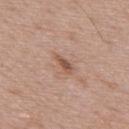automated_metrics:
  cielab_L: 53
  cielab_a: 20
  cielab_b: 29
  vs_skin_darker_L: 10.0
  vs_skin_contrast_norm: 7.5
lighting: white-light
site: upper back
patient:
  sex: male
  age_approx: 70
lesion_size:
  long_diameter_mm_approx: 2.5
image:
  source: total-body photography crop
  field_of_view_mm: 15